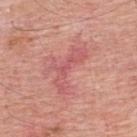Impression:
Recorded during total-body skin imaging; not selected for excision or biopsy.
Clinical summary:
Measured at roughly 6 mm in maximum diameter. The lesion is located on the upper back. The total-body-photography lesion software estimated a border-irregularity index near 8.5/10, a color-variation rating of about 1.5/10, and peripheral color asymmetry of about 0.5. Captured under white-light illumination. A close-up tile cropped from a whole-body skin photograph, about 15 mm across. A male patient approximately 60 years of age.